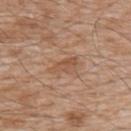{
  "biopsy_status": "not biopsied; imaged during a skin examination"
}